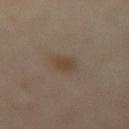{
  "lesion_size": {
    "long_diameter_mm_approx": 2.5
  },
  "patient": {
    "sex": "female",
    "age_approx": 55
  },
  "site": "lower back",
  "lighting": "cross-polarized",
  "image": {
    "source": "total-body photography crop",
    "field_of_view_mm": 15
  }
}